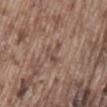Case summary:
- notes: no biopsy performed (imaged during a skin exam)
- automated lesion analysis: a mean CIELAB color near L≈48 a*≈16 b*≈24 and about 7 CIELAB-L* units darker than the surrounding skin; a color-variation rating of about 1.5/10 and radial color variation of about 0.5
- image source: ~15 mm crop, total-body skin-cancer survey
- diameter: about 3 mm
- location: the lower back
- subject: male, in their mid- to late 70s
- lighting: white-light illumination